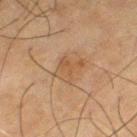– image · ~15 mm crop, total-body skin-cancer survey
– subject · male, aged approximately 65
– automated lesion analysis · an automated nevus-likeness rating near 5 out of 100
– lighting · cross-polarized
– lesion diameter · ~4 mm (longest diameter)
– site · the left thigh